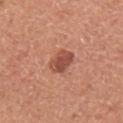The lesion was photographed on a routine skin check and not biopsied; there is no pathology result. The lesion is located on the right upper arm. A female subject, about 60 years old. A region of skin cropped from a whole-body photographic capture, roughly 15 mm wide. Captured under white-light illumination.